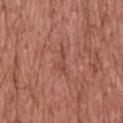| feature | finding |
|---|---|
| biopsy status | catalogued during a skin exam; not biopsied |
| image source | total-body-photography crop, ~15 mm field of view |
| subject | male, approximately 55 years of age |
| site | the back |
| TBP lesion metrics | an area of roughly 3.5 mm², an outline eccentricity of about 0.9 (0 = round, 1 = elongated), and two-axis asymmetry of about 0.55; a border-irregularity rating of about 6/10, a within-lesion color-variation index near 0.5/10, and a peripheral color-asymmetry measure near 0; a classifier nevus-likeness of about 0/100 |